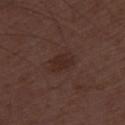Captured during whole-body skin photography for melanoma surveillance; the lesion was not biopsied. Measured at roughly 3.5 mm in maximum diameter. This is a white-light tile. A 15 mm close-up tile from a total-body photography series done for melanoma screening. The patient is a male approximately 50 years of age.